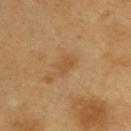subject: male, aged 58 to 62; image source: ~15 mm crop, total-body skin-cancer survey; anatomic site: the upper back.